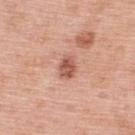Q: Was this lesion biopsied?
A: total-body-photography surveillance lesion; no biopsy